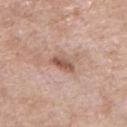<case>
<lesion_size>
  <long_diameter_mm_approx>3.0</long_diameter_mm_approx>
</lesion_size>
<patient>
  <sex>male</sex>
  <age_approx>60</age_approx>
</patient>
<lighting>white-light</lighting>
<image>
  <source>total-body photography crop</source>
  <field_of_view_mm>15</field_of_view_mm>
</image>
<site>chest</site>
<automated_metrics>
  <area_mm2_approx>4.0</area_mm2_approx>
  <eccentricity>0.85</eccentricity>
  <cielab_L>54</cielab_L>
  <cielab_a>20</cielab_a>
  <cielab_b>27</cielab_b>
  <vs_skin_contrast_norm>8.5</vs_skin_contrast_norm>
  <border_irregularity_0_10>2.5</border_irregularity_0_10>
  <peripheral_color_asymmetry>1.5</peripheral_color_asymmetry>
  <nevus_likeness_0_100>40</nevus_likeness_0_100>
  <lesion_detection_confidence_0_100>100</lesion_detection_confidence_0_100>
</automated_metrics>
</case>